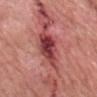* notes — no biopsy performed (imaged during a skin exam)
* image source — 15 mm crop, total-body photography
* body site — the head or neck
* subject — male, roughly 55 years of age
* lighting — white-light
* lesion diameter — ≈6 mm